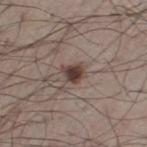{
  "biopsy_status": "not biopsied; imaged during a skin examination",
  "site": "left thigh",
  "image": {
    "source": "total-body photography crop",
    "field_of_view_mm": 15
  },
  "patient": {
    "sex": "male",
    "age_approx": 65
  }
}